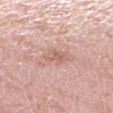This lesion was catalogued during total-body skin photography and was not selected for biopsy. The tile uses white-light illumination. On the leg. A 15 mm crop from a total-body photograph taken for skin-cancer surveillance. A female subject, approximately 40 years of age.